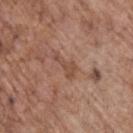No biopsy was performed on this lesion — it was imaged during a full skin examination and was not determined to be concerning. The tile uses white-light illumination. A male subject, approximately 70 years of age. The lesion's longest dimension is about 3.5 mm. This image is a 15 mm lesion crop taken from a total-body photograph. The lesion is on the upper back. The total-body-photography lesion software estimated a lesion area of about 4 mm², a shape eccentricity near 0.9, and a symmetry-axis asymmetry near 0.55. The software also gave a mean CIELAB color near L≈49 a*≈20 b*≈29, about 7 CIELAB-L* units darker than the surrounding skin, and a lesion-to-skin contrast of about 5.5 (normalized; higher = more distinct).